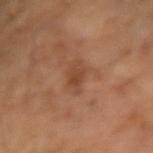Clinical summary: Cropped from a whole-body photographic skin survey; the tile spans about 15 mm. About 2.5 mm across. The tile uses cross-polarized illumination. A male subject aged 63 to 67. An algorithmic analysis of the crop reported a shape eccentricity near 0.75 and a shape-asymmetry score of about 0.3 (0 = symmetric). Located on the right forearm.